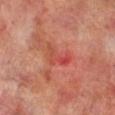Impression: Captured during whole-body skin photography for melanoma surveillance; the lesion was not biopsied. Clinical summary: Imaged with cross-polarized lighting. A 15 mm close-up extracted from a 3D total-body photography capture. Approximately 4 mm at its widest. A male subject, aged around 70. From the right lower leg.